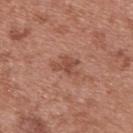workup: imaged on a skin check; not biopsied
image source: 15 mm crop, total-body photography
tile lighting: white-light
subject: female, in their 40s
site: the back